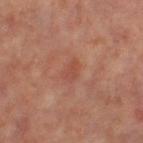<case>
<biopsy_status>not biopsied; imaged during a skin examination</biopsy_status>
<site>right leg</site>
<lesion_size>
  <long_diameter_mm_approx>2.5</long_diameter_mm_approx>
</lesion_size>
<patient>
  <sex>female</sex>
  <age_approx>65</age_approx>
</patient>
<automated_metrics>
  <area_mm2_approx>4.0</area_mm2_approx>
  <cielab_L>47</cielab_L>
  <cielab_a>25</cielab_a>
  <cielab_b>29</cielab_b>
  <vs_skin_contrast_norm>4.5</vs_skin_contrast_norm>
  <nevus_likeness_0_100>0</nevus_likeness_0_100>
  <lesion_detection_confidence_0_100>100</lesion_detection_confidence_0_100>
</automated_metrics>
<lighting>cross-polarized</lighting>
<image>
  <source>total-body photography crop</source>
  <field_of_view_mm>15</field_of_view_mm>
</image>
</case>